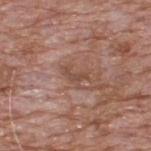Clinical impression: This lesion was catalogued during total-body skin photography and was not selected for biopsy. Background: On the upper back. Automated image analysis of the tile measured a classifier nevus-likeness of about 0/100. Cropped from a whole-body photographic skin survey; the tile spans about 15 mm. A male patient, about 60 years old. About 2.5 mm across.